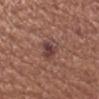lesion diameter = ≈3 mm | subject = male, in their mid-60s | image source = 15 mm crop, total-body photography | illumination = white-light | anatomic site = the left forearm.